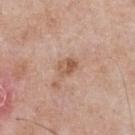Clinical impression:
No biopsy was performed on this lesion — it was imaged during a full skin examination and was not determined to be concerning.
Context:
This is a white-light tile. On the chest. The lesion's longest dimension is about 2.5 mm. The patient is a male in their 50s. A 15 mm crop from a total-body photograph taken for skin-cancer surveillance.